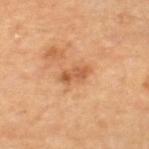follow-up: no biopsy performed (imaged during a skin exam) | diameter: ~3.5 mm (longest diameter) | image: ~15 mm tile from a whole-body skin photo | anatomic site: the right thigh | patient: male, aged 63–67 | tile lighting: cross-polarized.